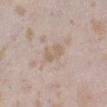Impression:
No biopsy was performed on this lesion — it was imaged during a full skin examination and was not determined to be concerning.
Clinical summary:
A 15 mm close-up tile from a total-body photography series done for melanoma screening. The total-body-photography lesion software estimated an area of roughly 4.5 mm² and a symmetry-axis asymmetry near 0.25. And it measured a mean CIELAB color near L≈62 a*≈14 b*≈26 and about 6 CIELAB-L* units darker than the surrounding skin. The analysis additionally found border irregularity of about 2.5 on a 0–10 scale and radial color variation of about 0.5. On the leg. The tile uses white-light illumination. A female patient, roughly 25 years of age. The lesion's longest dimension is about 3 mm.